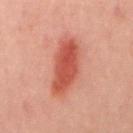Part of a total-body skin-imaging series; this lesion was reviewed on a skin check and was not flagged for biopsy.
Measured at roughly 7 mm in maximum diameter.
A close-up tile cropped from a whole-body skin photograph, about 15 mm across.
A male patient roughly 40 years of age.
On the mid back.